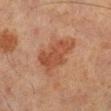• body site — the right lower leg
• TBP lesion metrics — a lesion color around L≈39 a*≈21 b*≈28 in CIELAB, about 8 CIELAB-L* units darker than the surrounding skin, and a normalized border contrast of about 7
• lighting — cross-polarized
• patient — male, about 65 years old
• image source — 15 mm crop, total-body photography